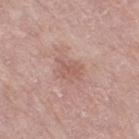Captured during whole-body skin photography for melanoma surveillance; the lesion was not biopsied. About 3 mm across. The patient is a female aged approximately 70. A roughly 15 mm field-of-view crop from a total-body skin photograph. The tile uses white-light illumination. The lesion-visualizer software estimated a lesion area of about 5 mm². The analysis additionally found a border-irregularity rating of about 4.5/10, a within-lesion color-variation index near 2/10, and radial color variation of about 0.5. On the leg.